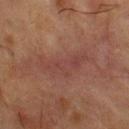<record>
  <biopsy_status>not biopsied; imaged during a skin examination</biopsy_status>
  <site>chest</site>
  <automated_metrics>
    <eccentricity>0.95</eccentricity>
    <shape_asymmetry>0.55</shape_asymmetry>
    <cielab_L>36</cielab_L>
    <cielab_a>20</cielab_a>
    <cielab_b>22</cielab_b>
    <vs_skin_darker_L>5.0</vs_skin_darker_L>
    <vs_skin_contrast_norm>5.0</vs_skin_contrast_norm>
    <nevus_likeness_0_100>0</nevus_likeness_0_100>
    <lesion_detection_confidence_0_100>85</lesion_detection_confidence_0_100>
  </automated_metrics>
  <image>
    <source>total-body photography crop</source>
    <field_of_view_mm>15</field_of_view_mm>
  </image>
  <patient>
    <sex>male</sex>
    <age_approx>55</age_approx>
  </patient>
  <lesion_size>
    <long_diameter_mm_approx>7.0</long_diameter_mm_approx>
  </lesion_size>
  <lighting>cross-polarized</lighting>
</record>